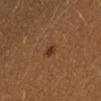A lesion tile, about 15 mm wide, cut from a 3D total-body photograph. From the left forearm. A female patient aged 28 to 32.